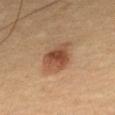Q: Was a biopsy performed?
A: no biopsy performed (imaged during a skin exam)
Q: What lighting was used for the tile?
A: cross-polarized illumination
Q: How was this image acquired?
A: total-body-photography crop, ~15 mm field of view
Q: Patient demographics?
A: male, about 65 years old
Q: What is the anatomic site?
A: the upper back
Q: How large is the lesion?
A: ≈4.5 mm